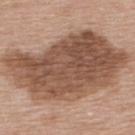Notes:
* workup: catalogued during a skin exam; not biopsied
* tile lighting: white-light
* TBP lesion metrics: an average lesion color of about L≈52 a*≈19 b*≈28 (CIELAB) and a lesion–skin lightness drop of about 14
* acquisition: ~15 mm crop, total-body skin-cancer survey
* subject: female, aged 38 to 42
* site: the back
* size: ≈14.5 mm